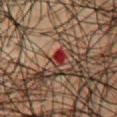biopsy status = imaged on a skin check; not biopsied
subject = male, in their mid-40s
automated lesion analysis = an area of roughly 3.5 mm², a shape eccentricity near 0.8, and two-axis asymmetry of about 0.25; a lesion color around L≈33 a*≈35 b*≈28 in CIELAB, a lesion–skin lightness drop of about 11, and a normalized lesion–skin contrast near 10.5; a border-irregularity index near 2.5/10, a color-variation rating of about 9/10, and a peripheral color-asymmetry measure near 3; a classifier nevus-likeness of about 0/100 and lesion-presence confidence of about 100/100
lighting = cross-polarized illumination
site = the chest
image source = ~15 mm crop, total-body skin-cancer survey